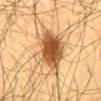{
  "biopsy_status": "not biopsied; imaged during a skin examination",
  "image": {
    "source": "total-body photography crop",
    "field_of_view_mm": 15
  },
  "patient": {
    "sex": "male",
    "age_approx": 60
  },
  "site": "front of the torso"
}